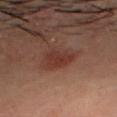Part of a total-body skin-imaging series; this lesion was reviewed on a skin check and was not flagged for biopsy. A close-up tile cropped from a whole-body skin photograph, about 15 mm across. A male subject, about 30 years old. From the head or neck.